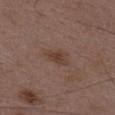The lesion was photographed on a routine skin check and not biopsied; there is no pathology result.